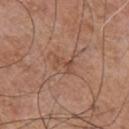<case>
  <biopsy_status>not biopsied; imaged during a skin examination</biopsy_status>
  <site>chest</site>
  <patient>
    <sex>male</sex>
    <age_approx>55</age_approx>
  </patient>
  <automated_metrics>
    <area_mm2_approx>2.5</area_mm2_approx>
    <eccentricity>0.85</eccentricity>
    <shape_asymmetry>0.45</shape_asymmetry>
    <lesion_detection_confidence_0_100>95</lesion_detection_confidence_0_100>
  </automated_metrics>
  <image>
    <source>total-body photography crop</source>
    <field_of_view_mm>15</field_of_view_mm>
  </image>
  <lesion_size>
    <long_diameter_mm_approx>2.5</long_diameter_mm_approx>
  </lesion_size>
  <lighting>white-light</lighting>
</case>